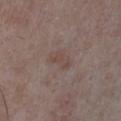A male subject, about 65 years old. This is a white-light tile. Cropped from a whole-body photographic skin survey; the tile spans about 15 mm. An algorithmic analysis of the crop reported a lesion area of about 3 mm², a shape eccentricity near 0.85, and two-axis asymmetry of about 0.4. And it measured about 5 CIELAB-L* units darker than the surrounding skin and a normalized lesion–skin contrast near 5. The software also gave border irregularity of about 4.5 on a 0–10 scale, a color-variation rating of about 0/10, and a peripheral color-asymmetry measure near 0. The software also gave a nevus-likeness score of about 0/100 and a detector confidence of about 100 out of 100 that the crop contains a lesion. The lesion is on the chest.